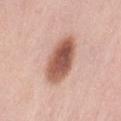biopsy status: no biopsy performed (imaged during a skin exam); body site: the left thigh; subject: female, aged approximately 50; image: 15 mm crop, total-body photography; illumination: white-light illumination.